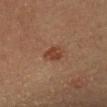– follow-up: imaged on a skin check; not biopsied
– diameter: ≈3 mm
– acquisition: total-body-photography crop, ~15 mm field of view
– illumination: cross-polarized illumination
– location: the head or neck
– subject: female, roughly 40 years of age